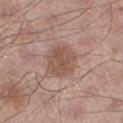Impression:
The lesion was tiled from a total-body skin photograph and was not biopsied.
Acquisition and patient details:
Cropped from a whole-body photographic skin survey; the tile spans about 15 mm. The lesion is located on the left lower leg. A male subject aged 53 to 57. The lesion's longest dimension is about 4.5 mm. The tile uses white-light illumination.